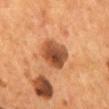Impression:
Part of a total-body skin-imaging series; this lesion was reviewed on a skin check and was not flagged for biopsy.
Context:
Cropped from a total-body skin-imaging series; the visible field is about 15 mm. An algorithmic analysis of the crop reported an area of roughly 12 mm² and a symmetry-axis asymmetry near 0.15. The software also gave a color-variation rating of about 6.5/10 and peripheral color asymmetry of about 2. The analysis additionally found a nevus-likeness score of about 50/100 and a lesion-detection confidence of about 100/100. Approximately 4.5 mm at its widest. Captured under cross-polarized illumination. A male subject, in their mid- to late 50s. From the mid back.